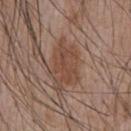Case summary:
- biopsy status: no biopsy performed (imaged during a skin exam)
- size: about 4.5 mm
- patient: male, aged approximately 55
- body site: the front of the torso
- automated metrics: a lesion color around L≈45 a*≈20 b*≈27 in CIELAB, a lesion–skin lightness drop of about 7, and a normalized lesion–skin contrast near 6; a nevus-likeness score of about 95/100 and a detector confidence of about 100 out of 100 that the crop contains a lesion
- image source: total-body-photography crop, ~15 mm field of view